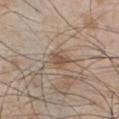biopsy status = catalogued during a skin exam; not biopsied | image = 15 mm crop, total-body photography | site = the chest | illumination = white-light illumination | patient = male, roughly 50 years of age | lesion diameter = ~2.5 mm (longest diameter) | automated metrics = a nevus-likeness score of about 15/100 and lesion-presence confidence of about 100/100.